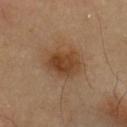Recorded during total-body skin imaging; not selected for excision or biopsy.
A region of skin cropped from a whole-body photographic capture, roughly 15 mm wide.
The lesion is located on the chest.